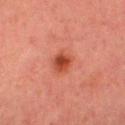follow-up: catalogued during a skin exam; not biopsied
image source: 15 mm crop, total-body photography
lighting: cross-polarized
automated lesion analysis: a lesion color around L≈42 a*≈31 b*≈32 in CIELAB, roughly 11 lightness units darker than nearby skin, and a normalized lesion–skin contrast near 9; a border-irregularity index near 2/10 and a within-lesion color-variation index near 4/10; an automated nevus-likeness rating near 100 out of 100 and a lesion-detection confidence of about 100/100
size: ≈3 mm
patient: female, roughly 70 years of age
anatomic site: the left thigh